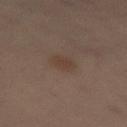Clinical impression: No biopsy was performed on this lesion — it was imaged during a full skin examination and was not determined to be concerning. Background: The subject is a male roughly 60 years of age. On the right thigh. A 15 mm crop from a total-body photograph taken for skin-cancer surveillance.